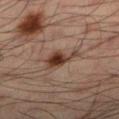• anatomic site — the left lower leg
• tile lighting — cross-polarized
• subject — male, in their mid-30s
• lesion diameter — ~4 mm (longest diameter)
• image source — ~15 mm tile from a whole-body skin photo